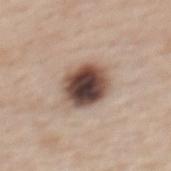Clinical impression: Captured during whole-body skin photography for melanoma surveillance; the lesion was not biopsied.